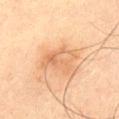biopsy status: imaged on a skin check; not biopsied | subject: male, approximately 60 years of age | image: total-body-photography crop, ~15 mm field of view | location: the abdomen | automated metrics: an outline eccentricity of about 0.7 (0 = round, 1 = elongated) and two-axis asymmetry of about 0.45; a lesion color around L≈56 a*≈19 b*≈33 in CIELAB and about 7 CIELAB-L* units darker than the surrounding skin; a border-irregularity rating of about 5/10, internal color variation of about 5.5 on a 0–10 scale, and peripheral color asymmetry of about 2 | lesion size: ~4 mm (longest diameter) | tile lighting: cross-polarized.